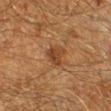Clinical impression: Imaged during a routine full-body skin examination; the lesion was not biopsied and no histopathology is available. Image and clinical context: This is a cross-polarized tile. The lesion is located on the right lower leg. A male patient, roughly 60 years of age. An algorithmic analysis of the crop reported an eccentricity of roughly 0.45. The analysis additionally found a lesion-to-skin contrast of about 7 (normalized; higher = more distinct). A 15 mm close-up tile from a total-body photography series done for melanoma screening.